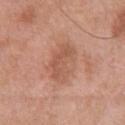Background:
The lesion is located on the front of the torso. The patient is a male roughly 70 years of age. A 15 mm close-up tile from a total-body photography series done for melanoma screening. The tile uses white-light illumination. The lesion-visualizer software estimated a lesion color around L≈56 a*≈23 b*≈31 in CIELAB and a normalized lesion–skin contrast near 6. And it measured lesion-presence confidence of about 100/100. The recorded lesion diameter is about 5 mm.